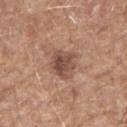  biopsy_status: not biopsied; imaged during a skin examination
  lesion_size:
    long_diameter_mm_approx: 4.0
  lighting: white-light
  automated_metrics:
    area_mm2_approx: 8.5
    eccentricity: 0.7
    shape_asymmetry: 0.3
    vs_skin_darker_L: 12.0
    vs_skin_contrast_norm: 8.5
    border_irregularity_0_10: 3.5
  site: right forearm
  patient:
    sex: male
    age_approx: 60
  image:
    source: total-body photography crop
    field_of_view_mm: 15